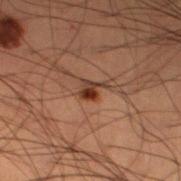• follow-up · total-body-photography surveillance lesion; no biopsy
• size · about 2.5 mm
• automated metrics · an average lesion color of about L≈29 a*≈19 b*≈25 (CIELAB), a lesion–skin lightness drop of about 11, and a normalized lesion–skin contrast near 10.5
• tile lighting · cross-polarized illumination
• patient · male, about 50 years old
• body site · the left thigh
• acquisition · ~15 mm tile from a whole-body skin photo